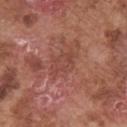* follow-up: total-body-photography surveillance lesion; no biopsy
* lesion size: ~3.5 mm (longest diameter)
* lighting: white-light illumination
* anatomic site: the right upper arm
* subject: male, approximately 75 years of age
* acquisition: total-body-photography crop, ~15 mm field of view